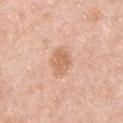Captured during whole-body skin photography for melanoma surveillance; the lesion was not biopsied. Imaged with white-light lighting. The lesion is located on the upper back. A 15 mm crop from a total-body photograph taken for skin-cancer surveillance. The subject is a male in their mid- to late 40s. The lesion's longest dimension is about 3.5 mm.